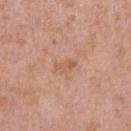Impression: Part of a total-body skin-imaging series; this lesion was reviewed on a skin check and was not flagged for biopsy. Background: The patient is a female roughly 40 years of age. Imaged with white-light lighting. This image is a 15 mm lesion crop taken from a total-body photograph. The lesion is on the right lower leg.